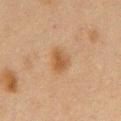Assessment: This lesion was catalogued during total-body skin photography and was not selected for biopsy. Clinical summary: The subject is a female aged approximately 40. Cropped from a total-body skin-imaging series; the visible field is about 15 mm. The total-body-photography lesion software estimated a footprint of about 5.5 mm² and an outline eccentricity of about 0.65 (0 = round, 1 = elongated). It also reported an automated nevus-likeness rating near 80 out of 100. Measured at roughly 3 mm in maximum diameter. On the chest.